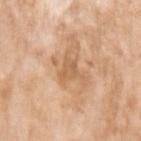Q: Is there a histopathology result?
A: imaged on a skin check; not biopsied
Q: Patient demographics?
A: female, in their mid- to late 70s
Q: How was this image acquired?
A: ~15 mm crop, total-body skin-cancer survey
Q: Lesion size?
A: ~3.5 mm (longest diameter)
Q: What is the anatomic site?
A: the arm